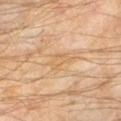Part of a total-body skin-imaging series; this lesion was reviewed on a skin check and was not flagged for biopsy.
On the left lower leg.
About 3 mm across.
This is a cross-polarized tile.
The patient is a male about 60 years old.
A roughly 15 mm field-of-view crop from a total-body skin photograph.